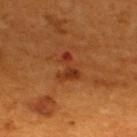The lesion was photographed on a routine skin check and not biopsied; there is no pathology result. Located on the upper back. About 3.5 mm across. The tile uses cross-polarized illumination. The patient is a male aged 58–62. A region of skin cropped from a whole-body photographic capture, roughly 15 mm wide.